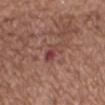| key | value |
|---|---|
| biopsy status | total-body-photography surveillance lesion; no biopsy |
| patient | female, roughly 75 years of age |
| automated metrics | roughly 7 lightness units darker than nearby skin and a normalized lesion–skin contrast near 6; border irregularity of about 5.5 on a 0–10 scale, internal color variation of about 6 on a 0–10 scale, and a peripheral color-asymmetry measure near 2 |
| body site | the mid back |
| diameter | ~3.5 mm (longest diameter) |
| imaging modality | ~15 mm crop, total-body skin-cancer survey |
| lighting | white-light |